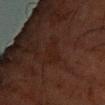Impression:
The lesion was tiled from a total-body skin photograph and was not biopsied.
Acquisition and patient details:
The lesion is located on the right forearm. A 15 mm close-up tile from a total-body photography series done for melanoma screening. The subject is a male aged approximately 65. About 3.5 mm across. The total-body-photography lesion software estimated border irregularity of about 4 on a 0–10 scale, a color-variation rating of about 1/10, and a peripheral color-asymmetry measure near 0.5. The analysis additionally found a detector confidence of about 100 out of 100 that the crop contains a lesion. Imaged with cross-polarized lighting.